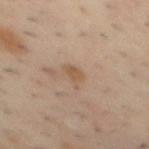Q: What kind of image is this?
A: 15 mm crop, total-body photography
Q: Where on the body is the lesion?
A: the back
Q: Who is the patient?
A: male, approximately 40 years of age